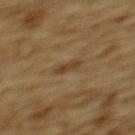Impression:
Captured during whole-body skin photography for melanoma surveillance; the lesion was not biopsied.
Background:
A lesion tile, about 15 mm wide, cut from a 3D total-body photograph. Captured under cross-polarized illumination. From the upper back. A male patient aged 83 or over.